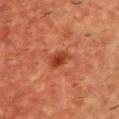Notes:
- follow-up · total-body-photography surveillance lesion; no biopsy
- illumination · cross-polarized illumination
- body site · the upper back
- subject · male, in their mid- to late 50s
- acquisition · total-body-photography crop, ~15 mm field of view
- lesion size · ≈3 mm
- automated metrics · a mean CIELAB color near L≈37 a*≈30 b*≈34 and about 10 CIELAB-L* units darker than the surrounding skin; a border-irregularity rating of about 2/10 and a color-variation rating of about 3.5/10